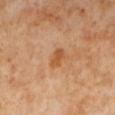biopsy status: imaged on a skin check; not biopsied | image source: ~15 mm tile from a whole-body skin photo | automated metrics: a footprint of about 3.5 mm² and two-axis asymmetry of about 0.3; a classifier nevus-likeness of about 25/100 | size: ~3 mm (longest diameter) | illumination: cross-polarized illumination | subject: female, about 50 years old | site: the left lower leg.